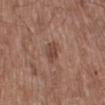<record>
  <biopsy_status>not biopsied; imaged during a skin examination</biopsy_status>
  <lighting>white-light</lighting>
  <image>
    <source>total-body photography crop</source>
    <field_of_view_mm>15</field_of_view_mm>
  </image>
  <lesion_size>
    <long_diameter_mm_approx>3.5</long_diameter_mm_approx>
  </lesion_size>
  <patient>
    <sex>male</sex>
    <age_approx>65</age_approx>
  </patient>
  <site>right lower leg</site>
  <automated_metrics>
    <nevus_likeness_0_100>5</nevus_likeness_0_100>
    <lesion_detection_confidence_0_100>100</lesion_detection_confidence_0_100>
  </automated_metrics>
</record>